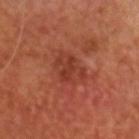Impression:
Part of a total-body skin-imaging series; this lesion was reviewed on a skin check and was not flagged for biopsy.
Acquisition and patient details:
The lesion is located on the head or neck. The recorded lesion diameter is about 4 mm. Cropped from a whole-body photographic skin survey; the tile spans about 15 mm. Captured under cross-polarized illumination. A male subject, aged approximately 65.